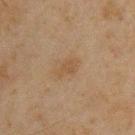Q: Was this lesion biopsied?
A: imaged on a skin check; not biopsied
Q: Where on the body is the lesion?
A: the chest
Q: How large is the lesion?
A: about 3 mm
Q: Patient demographics?
A: male, aged around 45
Q: How was this image acquired?
A: ~15 mm crop, total-body skin-cancer survey
Q: How was the tile lit?
A: cross-polarized illumination